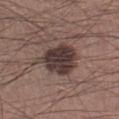Notes:
- biopsy status — catalogued during a skin exam; not biopsied
- location — the left lower leg
- patient — male, roughly 35 years of age
- size — ≈5.5 mm
- acquisition — ~15 mm crop, total-body skin-cancer survey
- tile lighting — white-light